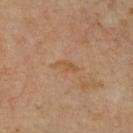Impression:
Captured during whole-body skin photography for melanoma surveillance; the lesion was not biopsied.
Acquisition and patient details:
The lesion's longest dimension is about 3 mm. Automated image analysis of the tile measured a lesion area of about 3 mm² and a shape eccentricity near 0.9. The analysis additionally found a classifier nevus-likeness of about 0/100. Imaged with cross-polarized lighting. On the right lower leg. A female subject aged 48 to 52. Cropped from a whole-body photographic skin survey; the tile spans about 15 mm.